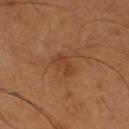lesion_size:
  long_diameter_mm_approx: 3.0
site: right thigh
patient:
  sex: male
  age_approx: 55
image:
  source: total-body photography crop
  field_of_view_mm: 15
lighting: cross-polarized